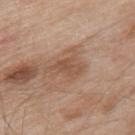biopsy_status: not biopsied; imaged during a skin examination
patient:
  sex: male
  age_approx: 55
image:
  source: total-body photography crop
  field_of_view_mm: 15
site: upper back
lighting: white-light
lesion_size:
  long_diameter_mm_approx: 4.5